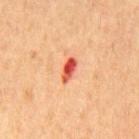Notes:
* biopsy status — catalogued during a skin exam; not biopsied
* subject — male, in their mid-60s
* illumination — cross-polarized
* body site — the mid back
* lesion size — ~3 mm (longest diameter)
* imaging modality — ~15 mm tile from a whole-body skin photo